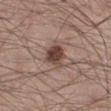{
  "biopsy_status": "not biopsied; imaged during a skin examination",
  "automated_metrics": {
    "cielab_L": 43,
    "cielab_a": 18,
    "cielab_b": 23,
    "vs_skin_darker_L": 14.0,
    "vs_skin_contrast_norm": 10.5
  },
  "lesion_size": {
    "long_diameter_mm_approx": 3.0
  },
  "image": {
    "source": "total-body photography crop",
    "field_of_view_mm": 15
  },
  "patient": {
    "sex": "male",
    "age_approx": 35
  },
  "site": "right lower leg",
  "lighting": "white-light"
}